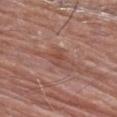Q: Is there a histopathology result?
A: imaged on a skin check; not biopsied
Q: How was this image acquired?
A: total-body-photography crop, ~15 mm field of view
Q: Patient demographics?
A: male, aged 78 to 82
Q: Lesion size?
A: about 3 mm
Q: Where on the body is the lesion?
A: the left upper arm